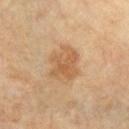Case summary:
* biopsy status — total-body-photography surveillance lesion; no biopsy
* diameter — ≈4.5 mm
* image source — 15 mm crop, total-body photography
* illumination — cross-polarized
* patient — male, in their 60s
* anatomic site — the chest